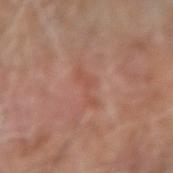biopsy status = no biopsy performed (imaged during a skin exam); image = ~15 mm crop, total-body skin-cancer survey; location = the left forearm; tile lighting = white-light; lesion size = ~4 mm (longest diameter); patient = male, aged approximately 75.